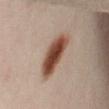biopsy status — no biopsy performed (imaged during a skin exam)
automated lesion analysis — an eccentricity of roughly 0.9 and a shape-asymmetry score of about 0.2 (0 = symmetric); a nevus-likeness score of about 100/100 and lesion-presence confidence of about 100/100
site — the left leg
diameter — about 6 mm
subject — female, about 40 years old
tile lighting — cross-polarized
image — ~15 mm tile from a whole-body skin photo